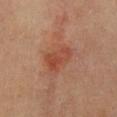Q: Was this lesion biopsied?
A: no biopsy performed (imaged during a skin exam)
Q: Patient demographics?
A: female, about 65 years old
Q: How was this image acquired?
A: ~15 mm tile from a whole-body skin photo
Q: Lesion size?
A: ≈4 mm
Q: How was the tile lit?
A: cross-polarized illumination
Q: Where on the body is the lesion?
A: the chest